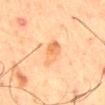This lesion was catalogued during total-body skin photography and was not selected for biopsy.
A close-up tile cropped from a whole-body skin photograph, about 15 mm across.
Located on the mid back.
A male subject, aged 58 to 62.